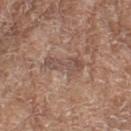image: total-body-photography crop, ~15 mm field of view | illumination: white-light illumination | subject: female, in their mid-70s | site: the leg.